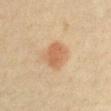Captured during whole-body skin photography for melanoma surveillance; the lesion was not biopsied.